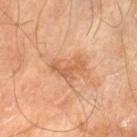The lesion was tiled from a total-body skin photograph and was not biopsied. Longest diameter approximately 4 mm. The subject is a male aged approximately 70. The tile uses cross-polarized illumination. The lesion is on the left thigh. A region of skin cropped from a whole-body photographic capture, roughly 15 mm wide. An algorithmic analysis of the crop reported a border-irregularity index near 8/10, a within-lesion color-variation index near 1/10, and a peripheral color-asymmetry measure near 0. The analysis additionally found an automated nevus-likeness rating near 0 out of 100.